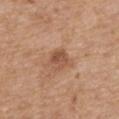The lesion was tiled from a total-body skin photograph and was not biopsied.
Captured under white-light illumination.
A roughly 15 mm field-of-view crop from a total-body skin photograph.
A male subject, aged around 70.
Automated tile analysis of the lesion measured an area of roughly 5.5 mm² and a symmetry-axis asymmetry near 0.2. And it measured a lesion color around L≈52 a*≈22 b*≈32 in CIELAB, roughly 10 lightness units darker than nearby skin, and a normalized lesion–skin contrast near 7. It also reported a border-irregularity rating of about 2/10 and radial color variation of about 1.5. It also reported an automated nevus-likeness rating near 0 out of 100 and lesion-presence confidence of about 100/100.
The lesion is located on the mid back.